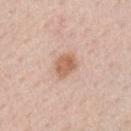workup — total-body-photography surveillance lesion; no biopsy | tile lighting — white-light | subject — male, aged 58–62 | body site — the right upper arm | image — total-body-photography crop, ~15 mm field of view | lesion size — ~3 mm (longest diameter).